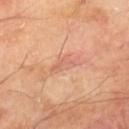| field | value |
|---|---|
| notes | total-body-photography surveillance lesion; no biopsy |
| imaging modality | ~15 mm tile from a whole-body skin photo |
| anatomic site | the left thigh |
| lesion diameter | ≈3.5 mm |
| patient | male, aged 68–72 |